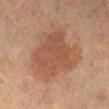Assessment: The lesion was tiled from a total-body skin photograph and was not biopsied. Context: A female subject, in their 60s. Measured at roughly 7 mm in maximum diameter. The total-body-photography lesion software estimated an eccentricity of roughly 0.5. It also reported a border-irregularity index near 3.5/10, a within-lesion color-variation index near 2.5/10, and peripheral color asymmetry of about 1. The software also gave a lesion-detection confidence of about 100/100. On the right lower leg. Captured under cross-polarized illumination. A 15 mm close-up tile from a total-body photography series done for melanoma screening.